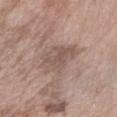Impression:
This lesion was catalogued during total-body skin photography and was not selected for biopsy.
Context:
The lesion is located on the right forearm. A 15 mm close-up extracted from a 3D total-body photography capture. A female patient, about 75 years old. Longest diameter approximately 4.5 mm.